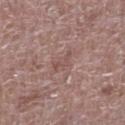The lesion was photographed on a routine skin check and not biopsied; there is no pathology result. Cropped from a total-body skin-imaging series; the visible field is about 15 mm. An algorithmic analysis of the crop reported an average lesion color of about L≈50 a*≈19 b*≈22 (CIELAB), roughly 6 lightness units darker than nearby skin, and a normalized lesion–skin contrast near 5. The analysis additionally found border irregularity of about 5.5 on a 0–10 scale, internal color variation of about 0 on a 0–10 scale, and radial color variation of about 0. On the leg. A male subject, aged around 70.